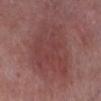Assessment:
Recorded during total-body skin imaging; not selected for excision or biopsy.
Background:
The total-body-photography lesion software estimated an outline eccentricity of about 0.6 (0 = round, 1 = elongated) and two-axis asymmetry of about 0.25. The lesion is located on the leg. About 7.5 mm across. A male subject aged 68–72. A region of skin cropped from a whole-body photographic capture, roughly 15 mm wide.